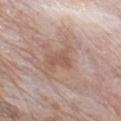notes = no biopsy performed (imaged during a skin exam) | location = the chest | illumination = white-light | image = total-body-photography crop, ~15 mm field of view | lesion diameter = ~2.5 mm (longest diameter) | patient = male, roughly 75 years of age.